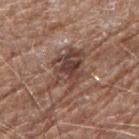Case summary:
– notes · no biopsy performed (imaged during a skin exam)
– anatomic site · the right forearm
– image source · ~15 mm crop, total-body skin-cancer survey
– patient · male, aged approximately 70